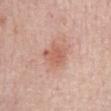notes = catalogued during a skin exam; not biopsied | imaging modality = ~15 mm tile from a whole-body skin photo | subject = female, about 65 years old | site = the abdomen.